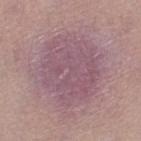<case>
<biopsy_status>not biopsied; imaged during a skin examination</biopsy_status>
<image>
  <source>total-body photography crop</source>
  <field_of_view_mm>15</field_of_view_mm>
</image>
<site>left thigh</site>
<automated_metrics>
  <area_mm2_approx>47.0</area_mm2_approx>
  <eccentricity>0.5</eccentricity>
  <border_irregularity_0_10>2.5</border_irregularity_0_10>
  <peripheral_color_asymmetry>1.0</peripheral_color_asymmetry>
</automated_metrics>
<patient>
  <sex>female</sex>
  <age_approx>40</age_approx>
</patient>
<lesion_size>
  <long_diameter_mm_approx>8.5</long_diameter_mm_approx>
</lesion_size>
</case>